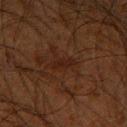<tbp_lesion>
<biopsy_status>not biopsied; imaged during a skin examination</biopsy_status>
<image>
  <source>total-body photography crop</source>
  <field_of_view_mm>15</field_of_view_mm>
</image>
<patient>
  <sex>male</sex>
  <age_approx>65</age_approx>
</patient>
<automated_metrics>
  <area_mm2_approx>2.5</area_mm2_approx>
  <eccentricity>0.95</eccentricity>
  <shape_asymmetry>0.3</shape_asymmetry>
  <border_irregularity_0_10>3.5</border_irregularity_0_10>
  <color_variation_0_10>0.0</color_variation_0_10>
  <peripheral_color_asymmetry>0.0</peripheral_color_asymmetry>
  <nevus_likeness_0_100>0</nevus_likeness_0_100>
  <lesion_detection_confidence_0_100>95</lesion_detection_confidence_0_100>
</automated_metrics>
<site>right upper arm</site>
<lighting>cross-polarized</lighting>
<lesion_size>
  <long_diameter_mm_approx>3.0</long_diameter_mm_approx>
</lesion_size>
</tbp_lesion>